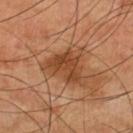Assessment:
Imaged during a routine full-body skin examination; the lesion was not biopsied and no histopathology is available.
Background:
A male subject, approximately 65 years of age. The total-body-photography lesion software estimated a shape-asymmetry score of about 0.5 (0 = symmetric). The software also gave peripheral color asymmetry of about 1.5. It also reported a classifier nevus-likeness of about 10/100 and lesion-presence confidence of about 100/100. On the left lower leg. This image is a 15 mm lesion crop taken from a total-body photograph.